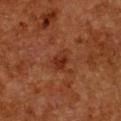biopsy status — total-body-photography surveillance lesion; no biopsy
size — about 3 mm
location — the chest
patient — female, in their 50s
imaging modality — 15 mm crop, total-body photography